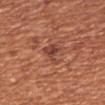Impression:
Captured during whole-body skin photography for melanoma surveillance; the lesion was not biopsied.
Acquisition and patient details:
A lesion tile, about 15 mm wide, cut from a 3D total-body photograph. The total-body-photography lesion software estimated border irregularity of about 5.5 on a 0–10 scale, internal color variation of about 3 on a 0–10 scale, and a peripheral color-asymmetry measure near 1. The analysis additionally found a nevus-likeness score of about 5/100 and a detector confidence of about 100 out of 100 that the crop contains a lesion. Measured at roughly 3 mm in maximum diameter. Located on the left arm. Imaged with white-light lighting. A female patient, aged 63 to 67.